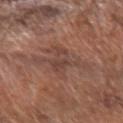Q: Was a biopsy performed?
A: total-body-photography surveillance lesion; no biopsy
Q: How large is the lesion?
A: about 5 mm
Q: Patient demographics?
A: male, about 70 years old
Q: What is the anatomic site?
A: the left forearm
Q: Automated lesion metrics?
A: a lesion area of about 14 mm², a shape eccentricity near 0.55, and a symmetry-axis asymmetry near 0.45; a mean CIELAB color near L≈44 a*≈20 b*≈24 and about 7 CIELAB-L* units darker than the surrounding skin
Q: What lighting was used for the tile?
A: white-light
Q: How was this image acquired?
A: ~15 mm tile from a whole-body skin photo